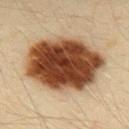This lesion was catalogued during total-body skin photography and was not selected for biopsy. Captured under cross-polarized illumination. A 15 mm close-up tile from a total-body photography series done for melanoma screening. An algorithmic analysis of the crop reported an outline eccentricity of about 0.7 (0 = round, 1 = elongated) and two-axis asymmetry of about 0.1. The analysis additionally found a normalized border contrast of about 17. The analysis additionally found a border-irregularity index near 1.5/10 and radial color variation of about 2. The software also gave a classifier nevus-likeness of about 100/100. The recorded lesion diameter is about 9 mm. The subject is a male aged 33 to 37. From the back.